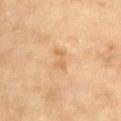Case summary:
- site: the chest
- subject: female, in their 50s
- illumination: cross-polarized
- diameter: about 3 mm
- imaging modality: total-body-photography crop, ~15 mm field of view